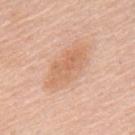Findings:
• notes · total-body-photography surveillance lesion; no biopsy
• body site · the back
• imaging modality · ~15 mm tile from a whole-body skin photo
• subject · male, about 60 years old